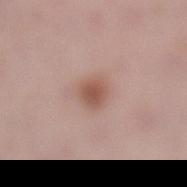Assessment:
Recorded during total-body skin imaging; not selected for excision or biopsy.